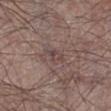{
  "image": {
    "source": "total-body photography crop",
    "field_of_view_mm": 15
  },
  "site": "leg",
  "lesion_size": {
    "long_diameter_mm_approx": 2.5
  },
  "lighting": "white-light",
  "patient": {
    "sex": "male",
    "age_approx": 65
  }
}